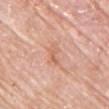Impression:
Captured during whole-body skin photography for melanoma surveillance; the lesion was not biopsied.
Clinical summary:
Cropped from a total-body skin-imaging series; the visible field is about 15 mm. The lesion is located on the chest. A female patient in their mid-60s. The total-body-photography lesion software estimated about 7 CIELAB-L* units darker than the surrounding skin and a normalized border contrast of about 5.5. The software also gave a border-irregularity index near 4.5/10, a color-variation rating of about 0/10, and radial color variation of about 0. The software also gave an automated nevus-likeness rating near 0 out of 100 and a detector confidence of about 100 out of 100 that the crop contains a lesion.